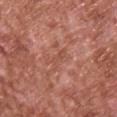Clinical impression: Part of a total-body skin-imaging series; this lesion was reviewed on a skin check and was not flagged for biopsy. Image and clinical context: A close-up tile cropped from a whole-body skin photograph, about 15 mm across. A male subject aged 63–67. About 3.5 mm across. Located on the upper back. Automated image analysis of the tile measured a mean CIELAB color near L≈51 a*≈26 b*≈30, roughly 6 lightness units darker than nearby skin, and a normalized lesion–skin contrast near 4.5. It also reported border irregularity of about 6 on a 0–10 scale, a within-lesion color-variation index near 0/10, and a peripheral color-asymmetry measure near 0.